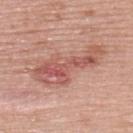Context: This image is a 15 mm lesion crop taken from a total-body photograph. The lesion is located on the back. Longest diameter approximately 9 mm. The subject is a female aged 58–62. Captured under white-light illumination.